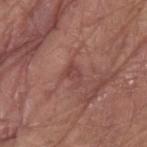<case>
  <biopsy_status>not biopsied; imaged during a skin examination</biopsy_status>
  <patient>
    <sex>male</sex>
    <age_approx>80</age_approx>
  </patient>
  <lesion_size>
    <long_diameter_mm_approx>2.5</long_diameter_mm_approx>
  </lesion_size>
  <lighting>white-light</lighting>
  <site>arm</site>
  <image>
    <source>total-body photography crop</source>
    <field_of_view_mm>15</field_of_view_mm>
  </image>
</case>